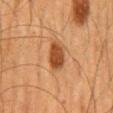Recorded during total-body skin imaging; not selected for excision or biopsy. A male subject aged approximately 65. The tile uses cross-polarized illumination. Located on the mid back. About 3.5 mm across. A 15 mm close-up extracted from a 3D total-body photography capture.